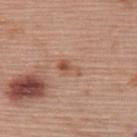The lesion was photographed on a routine skin check and not biopsied; there is no pathology result. This is a white-light tile. A female subject, aged approximately 60. Measured at roughly 2.5 mm in maximum diameter. On the upper back. A 15 mm close-up tile from a total-body photography series done for melanoma screening.